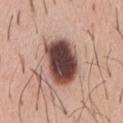Clinical impression:
Recorded during total-body skin imaging; not selected for excision or biopsy.
Image and clinical context:
The lesion is on the chest. The lesion-visualizer software estimated an outline eccentricity of about 0.75 (0 = round, 1 = elongated) and a symmetry-axis asymmetry near 0.1. And it measured a border-irregularity rating of about 1.5/10 and peripheral color asymmetry of about 2. The tile uses white-light illumination. A region of skin cropped from a whole-body photographic capture, roughly 15 mm wide. Approximately 6 mm at its widest. A male subject about 40 years old.